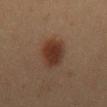| key | value |
|---|---|
| workup | imaged on a skin check; not biopsied |
| image source | 15 mm crop, total-body photography |
| anatomic site | the back |
| subject | female, aged around 20 |